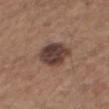  patient:
    sex: male
    age_approx: 65
  image:
    source: total-body photography crop
    field_of_view_mm: 15
  site: right thigh
  lighting: white-light
  lesion_size:
    long_diameter_mm_approx: 4.5